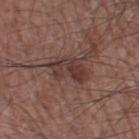Recorded during total-body skin imaging; not selected for excision or biopsy. A male subject aged 58 to 62. A roughly 15 mm field-of-view crop from a total-body skin photograph. This is a white-light tile. Automated tile analysis of the lesion measured a footprint of about 8.5 mm² and a shape eccentricity near 0.85. The analysis additionally found a lesion color around L≈36 a*≈18 b*≈20 in CIELAB and a normalized lesion–skin contrast near 8. On the left thigh. The recorded lesion diameter is about 4.5 mm.